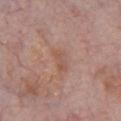No biopsy was performed on this lesion — it was imaged during a full skin examination and was not determined to be concerning. Imaged with white-light lighting. The lesion is on the chest. A 15 mm crop from a total-body photograph taken for skin-cancer surveillance. Measured at roughly 3 mm in maximum diameter. The subject is a male about 60 years old.